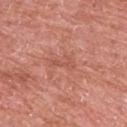follow-up: no biopsy performed (imaged during a skin exam) | subject: male, aged approximately 80 | image: total-body-photography crop, ~15 mm field of view | automated metrics: an average lesion color of about L≈54 a*≈28 b*≈30 (CIELAB) and about 6 CIELAB-L* units darker than the surrounding skin; a classifier nevus-likeness of about 0/100 and a detector confidence of about 100 out of 100 that the crop contains a lesion | lighting: white-light | location: the left upper arm | diameter: about 3 mm.